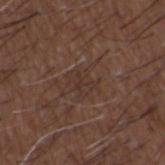lesion diameter: ~3 mm (longest diameter) | image: 15 mm crop, total-body photography | anatomic site: the upper back | tile lighting: white-light | patient: male, aged approximately 50.